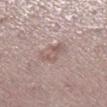• follow-up — catalogued during a skin exam; not biopsied